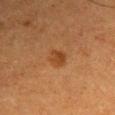notes = no biopsy performed (imaged during a skin exam)